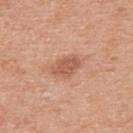Notes:
- biopsy status · imaged on a skin check; not biopsied
- body site · the upper back
- lesion size · ≈3.5 mm
- image-analysis metrics · a border-irregularity rating of about 2/10, a within-lesion color-variation index near 2/10, and a peripheral color-asymmetry measure near 0.5
- image source · ~15 mm crop, total-body skin-cancer survey
- lighting · white-light illumination
- subject · male, aged approximately 55